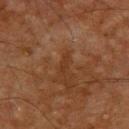Imaged with cross-polarized lighting.
A male patient aged around 65.
From the upper back.
A close-up tile cropped from a whole-body skin photograph, about 15 mm across.